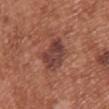notes — total-body-photography surveillance lesion; no biopsy | patient — female, approximately 40 years of age | imaging modality — total-body-photography crop, ~15 mm field of view | anatomic site — the upper back.